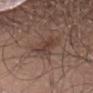Assessment: This lesion was catalogued during total-body skin photography and was not selected for biopsy. Background: An algorithmic analysis of the crop reported a lesion color around L≈40 a*≈17 b*≈23 in CIELAB, roughly 8 lightness units darker than nearby skin, and a normalized lesion–skin contrast near 6.5. It also reported border irregularity of about 5 on a 0–10 scale, a color-variation rating of about 3/10, and radial color variation of about 1. The lesion is on the abdomen. Approximately 5 mm at its widest. A 15 mm close-up extracted from a 3D total-body photography capture. The subject is a male in their 30s. The tile uses white-light illumination.